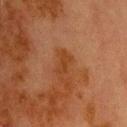Recorded during total-body skin imaging; not selected for excision or biopsy.
Imaged with cross-polarized lighting.
The lesion is located on the head or neck.
The lesion's longest dimension is about 3 mm.
A male patient, about 70 years old.
Cropped from a whole-body photographic skin survey; the tile spans about 15 mm.